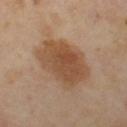Part of a total-body skin-imaging series; this lesion was reviewed on a skin check and was not flagged for biopsy.
A roughly 15 mm field-of-view crop from a total-body skin photograph.
Imaged with cross-polarized lighting.
A female subject, aged 43–47.
The lesion-visualizer software estimated a footprint of about 25 mm², an outline eccentricity of about 0.8 (0 = round, 1 = elongated), and two-axis asymmetry of about 0.2. The software also gave roughly 10 lightness units darker than nearby skin. It also reported border irregularity of about 2.5 on a 0–10 scale and a peripheral color-asymmetry measure near 1. The analysis additionally found a classifier nevus-likeness of about 80/100.
Measured at roughly 7 mm in maximum diameter.
From the left thigh.